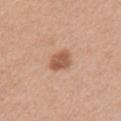Recorded during total-body skin imaging; not selected for excision or biopsy. Located on the left upper arm. A female subject roughly 35 years of age. A roughly 15 mm field-of-view crop from a total-body skin photograph.